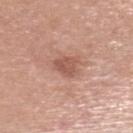Recorded during total-body skin imaging; not selected for excision or biopsy.
Imaged with white-light lighting.
About 3 mm across.
A lesion tile, about 15 mm wide, cut from a 3D total-body photograph.
The patient is a male roughly 70 years of age.
An algorithmic analysis of the crop reported a normalized lesion–skin contrast near 6.5. The analysis additionally found lesion-presence confidence of about 100/100.
From the head or neck.